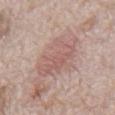Q: How was this image acquired?
A: total-body-photography crop, ~15 mm field of view
Q: Automated lesion metrics?
A: a border-irregularity rating of about 3.5/10, a within-lesion color-variation index near 3.5/10, and radial color variation of about 1
Q: What are the patient's age and sex?
A: male, aged 68–72
Q: Where on the body is the lesion?
A: the front of the torso